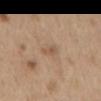Clinical impression:
Part of a total-body skin-imaging series; this lesion was reviewed on a skin check and was not flagged for biopsy.
Background:
The patient is a male aged approximately 70. The tile uses white-light illumination. From the mid back. An algorithmic analysis of the crop reported a border-irregularity index near 2.5/10, a color-variation rating of about 1.5/10, and peripheral color asymmetry of about 0.5. And it measured an automated nevus-likeness rating near 0 out of 100 and lesion-presence confidence of about 100/100. This image is a 15 mm lesion crop taken from a total-body photograph. Measured at roughly 2.5 mm in maximum diameter.